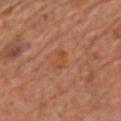Q: Is there a histopathology result?
A: imaged on a skin check; not biopsied
Q: What is the anatomic site?
A: the front of the torso
Q: What kind of image is this?
A: 15 mm crop, total-body photography
Q: Automated lesion metrics?
A: a border-irregularity index near 4/10 and a within-lesion color-variation index near 0.5/10; a nevus-likeness score of about 0/100 and lesion-presence confidence of about 100/100
Q: Lesion size?
A: ~2.5 mm (longest diameter)
Q: What lighting was used for the tile?
A: cross-polarized illumination
Q: Who is the patient?
A: female, aged 58–62